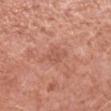Q: Was this lesion biopsied?
A: total-body-photography surveillance lesion; no biopsy
Q: What lighting was used for the tile?
A: white-light
Q: Where on the body is the lesion?
A: the left forearm
Q: What is the lesion's diameter?
A: ≈3.5 mm
Q: Patient demographics?
A: female, approximately 40 years of age
Q: What kind of image is this?
A: 15 mm crop, total-body photography